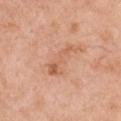<tbp_lesion>
<biopsy_status>not biopsied; imaged during a skin examination</biopsy_status>
<lighting>white-light</lighting>
<image>
  <source>total-body photography crop</source>
  <field_of_view_mm>15</field_of_view_mm>
</image>
<site>chest</site>
<lesion_size>
  <long_diameter_mm_approx>5.0</long_diameter_mm_approx>
</lesion_size>
<patient>
  <sex>female</sex>
  <age_approx>55</age_approx>
</patient>
</tbp_lesion>